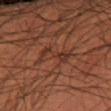| key | value |
|---|---|
| workup | total-body-photography surveillance lesion; no biopsy |
| automated metrics | a mean CIELAB color near L≈25 a*≈18 b*≈22, roughly 5 lightness units darker than nearby skin, and a normalized lesion–skin contrast near 5.5 |
| image | total-body-photography crop, ~15 mm field of view |
| patient | male, about 60 years old |
| tile lighting | cross-polarized |
| diameter | about 4.5 mm |
| body site | the right forearm |